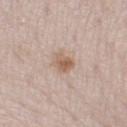| field | value |
|---|---|
| lighting | white-light |
| imaging modality | total-body-photography crop, ~15 mm field of view |
| body site | the right thigh |
| patient | male, aged approximately 80 |